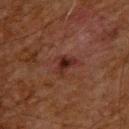Part of a total-body skin-imaging series; this lesion was reviewed on a skin check and was not flagged for biopsy.
On the upper back.
Approximately 3 mm at its widest.
A male patient in their 60s.
This image is a 15 mm lesion crop taken from a total-body photograph.
Captured under cross-polarized illumination.